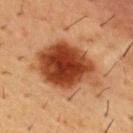notes — no biopsy performed (imaged during a skin exam); illumination — cross-polarized illumination; image — ~15 mm tile from a whole-body skin photo; lesion size — about 8 mm; patient — male, in their mid-50s; anatomic site — the front of the torso.